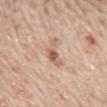Imaged during a routine full-body skin examination; the lesion was not biopsied and no histopathology is available.
A male subject in their mid-60s.
This is a white-light tile.
Located on the back.
Longest diameter approximately 4 mm.
A lesion tile, about 15 mm wide, cut from a 3D total-body photograph.